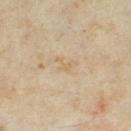Recorded during total-body skin imaging; not selected for excision or biopsy. A 15 mm close-up tile from a total-body photography series done for melanoma screening. A female patient in their mid- to late 30s. The lesion is located on the right thigh.